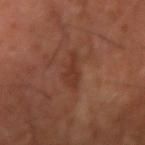notes: no biopsy performed (imaged during a skin exam) | patient: male, aged approximately 70 | anatomic site: the arm | imaging modality: ~15 mm crop, total-body skin-cancer survey | lesion size: ≈4 mm | image-analysis metrics: an area of roughly 4.5 mm², a shape eccentricity near 0.9, and two-axis asymmetry of about 0.45; a nevus-likeness score of about 0/100 and a lesion-detection confidence of about 100/100.